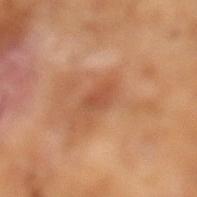Imaged during a routine full-body skin examination; the lesion was not biopsied and no histopathology is available. A male patient in their mid- to late 60s. Cropped from a whole-body photographic skin survey; the tile spans about 15 mm. About 2.5 mm across.